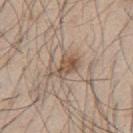Impression: Recorded during total-body skin imaging; not selected for excision or biopsy. Image and clinical context: From the mid back. A male patient about 45 years old. A roughly 15 mm field-of-view crop from a total-body skin photograph.